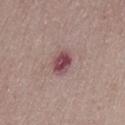Imaged during a routine full-body skin examination; the lesion was not biopsied and no histopathology is available. From the abdomen. A lesion tile, about 15 mm wide, cut from a 3D total-body photograph. The patient is a female aged 48 to 52. Measured at roughly 3 mm in maximum diameter. Automated image analysis of the tile measured an area of roughly 5.5 mm², an outline eccentricity of about 0.7 (0 = round, 1 = elongated), and two-axis asymmetry of about 0.2. It also reported a mean CIELAB color near L≈46 a*≈25 b*≈16, a lesion–skin lightness drop of about 14, and a normalized border contrast of about 10. The analysis additionally found an automated nevus-likeness rating near 0 out of 100 and lesion-presence confidence of about 100/100. Captured under white-light illumination.